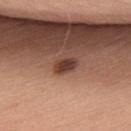Part of a total-body skin-imaging series; this lesion was reviewed on a skin check and was not flagged for biopsy. Cropped from a total-body skin-imaging series; the visible field is about 15 mm. Approximately 3 mm at its widest. The patient is a female about 45 years old. The lesion is located on the upper back. Automated image analysis of the tile measured a footprint of about 4 mm², a shape eccentricity near 0.8, and a symmetry-axis asymmetry near 0.15. And it measured a border-irregularity rating of about 1.5/10, internal color variation of about 3 on a 0–10 scale, and peripheral color asymmetry of about 1. The software also gave a classifier nevus-likeness of about 55/100. Imaged with white-light lighting.